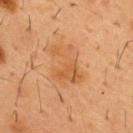follow-up = catalogued during a skin exam; not biopsied
patient = male, aged 53 to 57
anatomic site = the chest
imaging modality = ~15 mm tile from a whole-body skin photo